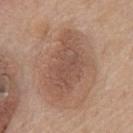The lesion was photographed on a routine skin check and not biopsied; there is no pathology result. A male patient, roughly 75 years of age. Imaged with white-light lighting. A 15 mm close-up tile from a total-body photography series done for melanoma screening. The lesion is on the front of the torso.